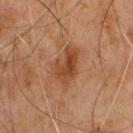<record>
  <biopsy_status>not biopsied; imaged during a skin examination</biopsy_status>
  <lesion_size>
    <long_diameter_mm_approx>4.5</long_diameter_mm_approx>
  </lesion_size>
  <lighting>cross-polarized</lighting>
  <patient>
    <sex>male</sex>
    <age_approx>65</age_approx>
  </patient>
  <automated_metrics>
    <area_mm2_approx>9.0</area_mm2_approx>
    <eccentricity>0.8</eccentricity>
    <shape_asymmetry>0.35</shape_asymmetry>
    <cielab_L>44</cielab_L>
    <cielab_a>24</cielab_a>
    <cielab_b>35</cielab_b>
    <vs_skin_darker_L>10.0</vs_skin_darker_L>
    <vs_skin_contrast_norm>8.0</vs_skin_contrast_norm>
    <nevus_likeness_0_100>60</nevus_likeness_0_100>
    <lesion_detection_confidence_0_100>100</lesion_detection_confidence_0_100>
  </automated_metrics>
  <image>
    <source>total-body photography crop</source>
    <field_of_view_mm>15</field_of_view_mm>
  </image>
</record>